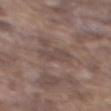Clinical impression: The lesion was tiled from a total-body skin photograph and was not biopsied. Acquisition and patient details: From the back. A lesion tile, about 15 mm wide, cut from a 3D total-body photograph. Imaged with white-light lighting. A male subject, aged 73 to 77. Automated tile analysis of the lesion measured a lesion–skin lightness drop of about 6. And it measured a lesion-detection confidence of about 70/100. Longest diameter approximately 3 mm.